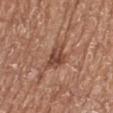No biopsy was performed on this lesion — it was imaged during a full skin examination and was not determined to be concerning.